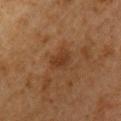The lesion was tiled from a total-body skin photograph and was not biopsied. From the chest. Longest diameter approximately 3 mm. A male patient, aged 58 to 62. The total-body-photography lesion software estimated an area of roughly 5 mm², an outline eccentricity of about 0.65 (0 = round, 1 = elongated), and a shape-asymmetry score of about 0.2 (0 = symmetric). It also reported an average lesion color of about L≈30 a*≈17 b*≈28 (CIELAB) and a lesion-to-skin contrast of about 6.5 (normalized; higher = more distinct). It also reported border irregularity of about 2 on a 0–10 scale, a color-variation rating of about 2/10, and radial color variation of about 0.5. And it measured a classifier nevus-likeness of about 5/100. The tile uses cross-polarized illumination. This image is a 15 mm lesion crop taken from a total-body photograph.